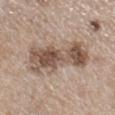No biopsy was performed on this lesion — it was imaged during a full skin examination and was not determined to be concerning. The lesion is on the right lower leg. Imaged with white-light lighting. Measured at roughly 8 mm in maximum diameter. A female patient, roughly 70 years of age. Automated tile analysis of the lesion measured a border-irregularity rating of about 5/10, a color-variation rating of about 7.5/10, and peripheral color asymmetry of about 3. The software also gave a nevus-likeness score of about 90/100 and a detector confidence of about 100 out of 100 that the crop contains a lesion. A 15 mm close-up extracted from a 3D total-body photography capture.